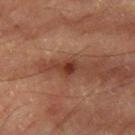Impression: Imaged during a routine full-body skin examination; the lesion was not biopsied and no histopathology is available. Clinical summary: A 15 mm close-up tile from a total-body photography series done for melanoma screening. On the right thigh. The subject is a male in their mid- to late 80s or older. Measured at roughly 2.5 mm in maximum diameter. Captured under cross-polarized illumination.